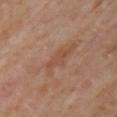Q: Was this lesion biopsied?
A: imaged on a skin check; not biopsied
Q: Patient demographics?
A: female, aged around 50
Q: Where on the body is the lesion?
A: the right upper arm
Q: How was this image acquired?
A: total-body-photography crop, ~15 mm field of view